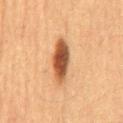Background:
Cropped from a total-body skin-imaging series; the visible field is about 15 mm. The patient is a male roughly 65 years of age. From the mid back.